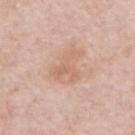Q: Was this lesion biopsied?
A: total-body-photography surveillance lesion; no biopsy
Q: What is the lesion's diameter?
A: ~4 mm (longest diameter)
Q: Patient demographics?
A: male, approximately 55 years of age
Q: How was the tile lit?
A: white-light
Q: How was this image acquired?
A: 15 mm crop, total-body photography
Q: What is the anatomic site?
A: the chest